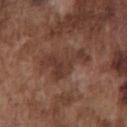{"biopsy_status": "not biopsied; imaged during a skin examination", "patient": {"sex": "male", "age_approx": 75}, "lighting": "white-light", "image": {"source": "total-body photography crop", "field_of_view_mm": 15}, "site": "chest", "lesion_size": {"long_diameter_mm_approx": 6.0}}